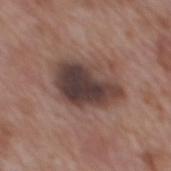  biopsy_status: not biopsied; imaged during a skin examination
  patient:
    sex: male
    age_approx: 70
  lighting: white-light
  lesion_size:
    long_diameter_mm_approx: 6.5
  image:
    source: total-body photography crop
    field_of_view_mm: 15
  site: mid back